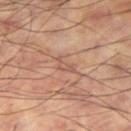This lesion was catalogued during total-body skin photography and was not selected for biopsy. Cropped from a total-body skin-imaging series; the visible field is about 15 mm. Measured at roughly 2.5 mm in maximum diameter. The lesion is on the right thigh. The lesion-visualizer software estimated an average lesion color of about L≈54 a*≈21 b*≈28 (CIELAB) and about 7 CIELAB-L* units darker than the surrounding skin. And it measured a border-irregularity rating of about 5.5/10 and a color-variation rating of about 0/10. The analysis additionally found a nevus-likeness score of about 0/100 and a lesion-detection confidence of about 55/100. The patient is a male about 60 years old.